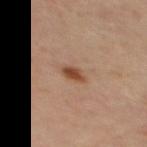image:
  source: total-body photography crop
  field_of_view_mm: 15
lighting: cross-polarized
lesion_size:
  long_diameter_mm_approx: 2.5
site: mid back
automated_metrics:
  cielab_L: 45
  cielab_a: 21
  cielab_b: 30
  vs_skin_darker_L: 11.0
  vs_skin_contrast_norm: 9.0
  border_irregularity_0_10: 2.5
  color_variation_0_10: 2.0
  peripheral_color_asymmetry: 1.0
  nevus_likeness_0_100: 95
  lesion_detection_confidence_0_100: 100
patient:
  sex: female
  age_approx: 45